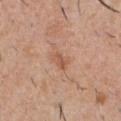Findings:
– follow-up — no biopsy performed (imaged during a skin exam)
– site — the chest
– patient — male, aged approximately 30
– acquisition — total-body-photography crop, ~15 mm field of view
– automated lesion analysis — a shape eccentricity near 0.8
– illumination — white-light illumination
– diameter — about 2.5 mm